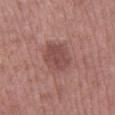Assessment: Part of a total-body skin-imaging series; this lesion was reviewed on a skin check and was not flagged for biopsy.